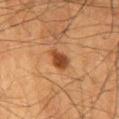Impression:
Imaged during a routine full-body skin examination; the lesion was not biopsied and no histopathology is available.
Acquisition and patient details:
A close-up tile cropped from a whole-body skin photograph, about 15 mm across. The lesion is on the mid back. A male subject, in their mid- to late 60s.